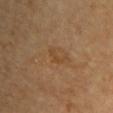Clinical impression: Imaged during a routine full-body skin examination; the lesion was not biopsied and no histopathology is available. Background: A male subject in their mid-80s or older. Approximately 3 mm at its widest. A 15 mm close-up tile from a total-body photography series done for melanoma screening. The lesion is located on the chest.